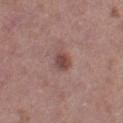Part of a total-body skin-imaging series; this lesion was reviewed on a skin check and was not flagged for biopsy. The subject is a female in their 50s. The total-body-photography lesion software estimated a footprint of about 4 mm² and a shape eccentricity near 0.7. And it measured internal color variation of about 3 on a 0–10 scale. Longest diameter approximately 2.5 mm. Captured under white-light illumination. On the left thigh. This image is a 15 mm lesion crop taken from a total-body photograph.